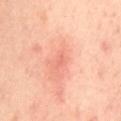workup: imaged on a skin check; not biopsied
imaging modality: ~15 mm crop, total-body skin-cancer survey
anatomic site: the mid back
lesion size: ≈1.5 mm
patient: female, in their 40s
TBP lesion metrics: an average lesion color of about L≈67 a*≈34 b*≈33 (CIELAB), about 7 CIELAB-L* units darker than the surrounding skin, and a lesion-to-skin contrast of about 4 (normalized; higher = more distinct)
illumination: cross-polarized